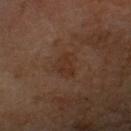<case>
  <biopsy_status>not biopsied; imaged during a skin examination</biopsy_status>
  <automated_metrics>
    <area_mm2_approx>6.5</area_mm2_approx>
    <shape_asymmetry>0.3</shape_asymmetry>
    <cielab_L>30</cielab_L>
    <cielab_a>17</cielab_a>
    <cielab_b>26</cielab_b>
    <vs_skin_darker_L>5.0</vs_skin_darker_L>
    <border_irregularity_0_10>2.5</border_irregularity_0_10>
    <peripheral_color_asymmetry>1.0</peripheral_color_asymmetry>
  </automated_metrics>
  <patient>
    <sex>male</sex>
    <age_approx>60</age_approx>
  </patient>
  <image>
    <source>total-body photography crop</source>
    <field_of_view_mm>15</field_of_view_mm>
  </image>
  <site>head or neck</site>
</case>